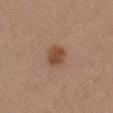Part of a total-body skin-imaging series; this lesion was reviewed on a skin check and was not flagged for biopsy.
A roughly 15 mm field-of-view crop from a total-body skin photograph.
The recorded lesion diameter is about 2.5 mm.
From the arm.
This is a white-light tile.
A female patient, in their 40s.